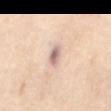{
  "biopsy_status": "not biopsied; imaged during a skin examination",
  "lighting": "cross-polarized",
  "image": {
    "source": "total-body photography crop",
    "field_of_view_mm": 15
  },
  "automated_metrics": {
    "vs_skin_darker_L": 14.0,
    "vs_skin_contrast_norm": 9.0,
    "nevus_likeness_0_100": 0,
    "lesion_detection_confidence_0_100": 60
  },
  "patient": {
    "sex": "female",
    "age_approx": 55
  },
  "site": "mid back",
  "lesion_size": {
    "long_diameter_mm_approx": 3.0
  }
}